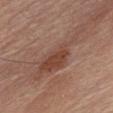follow-up = total-body-photography surveillance lesion; no biopsy
image source = total-body-photography crop, ~15 mm field of view
body site = the chest
subject = male, aged approximately 60